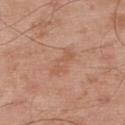follow-up = total-body-photography surveillance lesion; no biopsy | subject = male, roughly 55 years of age | image source = 15 mm crop, total-body photography | anatomic site = the upper back | illumination = white-light illumination | size = ≈3.5 mm.